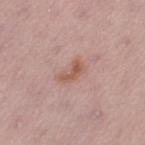{
  "biopsy_status": "not biopsied; imaged during a skin examination",
  "image": {
    "source": "total-body photography crop",
    "field_of_view_mm": 15
  },
  "lesion_size": {
    "long_diameter_mm_approx": 3.0
  },
  "site": "left thigh",
  "automated_metrics": {
    "area_mm2_approx": 4.0,
    "color_variation_0_10": 1.0,
    "nevus_likeness_0_100": 50,
    "lesion_detection_confidence_0_100": 100
  },
  "patient": {
    "sex": "female",
    "age_approx": 50
  }
}